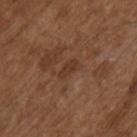biopsy_status: not biopsied; imaged during a skin examination
lighting: white-light
patient:
  sex: male
  age_approx: 75
image:
  source: total-body photography crop
  field_of_view_mm: 15
site: right upper arm
automated_metrics:
  area_mm2_approx: 3.0
  eccentricity: 0.85
  cielab_L: 35
  cielab_a: 19
  cielab_b: 27
  vs_skin_darker_L: 6.0
  border_irregularity_0_10: 2.5
  color_variation_0_10: 1.5
  peripheral_color_asymmetry: 0.5
  lesion_detection_confidence_0_100: 100
lesion_size:
  long_diameter_mm_approx: 2.5